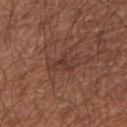Impression:
Imaged during a routine full-body skin examination; the lesion was not biopsied and no histopathology is available.
Image and clinical context:
A male subject, approximately 65 years of age. Cropped from a total-body skin-imaging series; the visible field is about 15 mm. The lesion is located on the right forearm. Automated tile analysis of the lesion measured an automated nevus-likeness rating near 0 out of 100 and a lesion-detection confidence of about 95/100. Captured under white-light illumination.